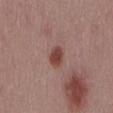Part of a total-body skin-imaging series; this lesion was reviewed on a skin check and was not flagged for biopsy.
Captured under white-light illumination.
A 15 mm close-up extracted from a 3D total-body photography capture.
Automated image analysis of the tile measured a footprint of about 4.5 mm², an eccentricity of roughly 0.75, and a shape-asymmetry score of about 0.2 (0 = symmetric). The software also gave a color-variation rating of about 2.5/10. The software also gave a lesion-detection confidence of about 100/100.
The lesion's longest dimension is about 3 mm.
A male patient about 55 years old.
The lesion is located on the back.